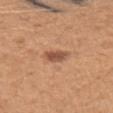<tbp_lesion>
  <biopsy_status>not biopsied; imaged during a skin examination</biopsy_status>
  <lighting>white-light</lighting>
  <image>
    <source>total-body photography crop</source>
    <field_of_view_mm>15</field_of_view_mm>
  </image>
  <site>arm</site>
  <lesion_size>
    <long_diameter_mm_approx>2.5</long_diameter_mm_approx>
  </lesion_size>
  <patient>
    <sex>female</sex>
    <age_approx>45</age_approx>
  </patient>
</tbp_lesion>